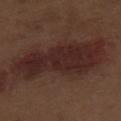<record>
<lighting>white-light</lighting>
<patient>
  <sex>male</sex>
  <age_approx>70</age_approx>
</patient>
<image>
  <source>total-body photography crop</source>
  <field_of_view_mm>15</field_of_view_mm>
</image>
<site>leg</site>
</record>